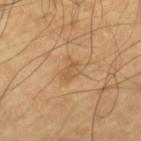Findings:
* workup · total-body-photography surveillance lesion; no biopsy
* lighting · cross-polarized
* image source · total-body-photography crop, ~15 mm field of view
* patient · male, in their mid- to late 60s
* body site · the right lower leg